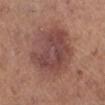Impression: This lesion was catalogued during total-body skin photography and was not selected for biopsy. Context: The recorded lesion diameter is about 7.5 mm. A 15 mm crop from a total-body photograph taken for skin-cancer surveillance. Imaged with white-light lighting. From the right lower leg. A female patient, approximately 65 years of age.